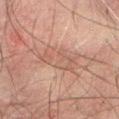Clinical impression:
The lesion was photographed on a routine skin check and not biopsied; there is no pathology result.
Image and clinical context:
A lesion tile, about 15 mm wide, cut from a 3D total-body photograph. Approximately 5 mm at its widest. A male patient aged 58 to 62. The lesion is on the abdomen.